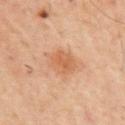Case summary:
- anatomic site: the upper back
- patient: male, aged approximately 60
- image source: total-body-photography crop, ~15 mm field of view